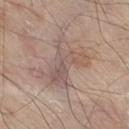| field | value |
|---|---|
| follow-up | total-body-photography surveillance lesion; no biopsy |
| lesion size | ~6.5 mm (longest diameter) |
| site | the right thigh |
| acquisition | ~15 mm crop, total-body skin-cancer survey |
| subject | male, roughly 80 years of age |
| illumination | white-light |